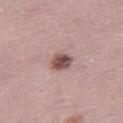Case summary:
- lighting — white-light illumination
- subject — female, in their 50s
- image — ~15 mm crop, total-body skin-cancer survey
- anatomic site — the right thigh
- lesion size — ≈2.5 mm